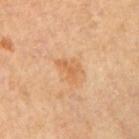{"biopsy_status": "not biopsied; imaged during a skin examination", "automated_metrics": {"nevus_likeness_0_100": 5}, "patient": {"sex": "male", "age_approx": 65}, "site": "arm", "image": {"source": "total-body photography crop", "field_of_view_mm": 15}}